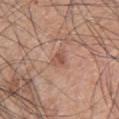{"biopsy_status": "not biopsied; imaged during a skin examination", "lighting": "white-light", "lesion_size": {"long_diameter_mm_approx": 2.5}, "patient": {"sex": "male", "age_approx": 70}, "image": {"source": "total-body photography crop", "field_of_view_mm": 15}, "site": "upper back"}